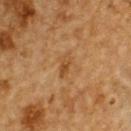– follow-up: total-body-photography surveillance lesion; no biopsy
– size: about 3 mm
– anatomic site: the upper back
– patient: male, approximately 85 years of age or older
– imaging modality: ~15 mm tile from a whole-body skin photo
– lighting: cross-polarized